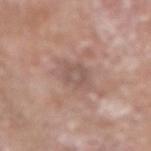Imaged during a routine full-body skin examination; the lesion was not biopsied and no histopathology is available.
Automated image analysis of the tile measured a lesion area of about 8 mm², a shape eccentricity near 0.55, and a symmetry-axis asymmetry near 0.2. And it measured a mean CIELAB color near L≈54 a*≈17 b*≈23, a lesion–skin lightness drop of about 7, and a normalized border contrast of about 5. The software also gave a nevus-likeness score of about 0/100 and a detector confidence of about 90 out of 100 that the crop contains a lesion.
Captured under white-light illumination.
Cropped from a whole-body photographic skin survey; the tile spans about 15 mm.
About 3.5 mm across.
The patient is a male aged around 80.
The lesion is on the left forearm.